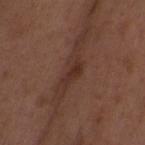<lesion>
  <site>back</site>
  <patient>
    <sex>female</sex>
    <age_approx>35</age_approx>
  </patient>
  <image>
    <source>total-body photography crop</source>
    <field_of_view_mm>15</field_of_view_mm>
  </image>
</lesion>